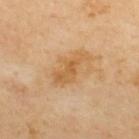Notes:
* biopsy status: catalogued during a skin exam; not biopsied
* patient: male, aged 53–57
* lighting: cross-polarized illumination
* anatomic site: the upper back
* automated metrics: roughly 8 lightness units darker than nearby skin and a normalized border contrast of about 6.5; a border-irregularity index near 5/10; a classifier nevus-likeness of about 0/100 and a detector confidence of about 100 out of 100 that the crop contains a lesion
* image source: total-body-photography crop, ~15 mm field of view